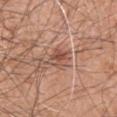Part of a total-body skin-imaging series; this lesion was reviewed on a skin check and was not flagged for biopsy. A 15 mm crop from a total-body photograph taken for skin-cancer surveillance. Automated tile analysis of the lesion measured a lesion area of about 5 mm² and an outline eccentricity of about 0.5 (0 = round, 1 = elongated). And it measured a lesion-to-skin contrast of about 6.5 (normalized; higher = more distinct). The lesion's longest dimension is about 2.5 mm. A male patient, aged 58–62. Located on the left upper arm.